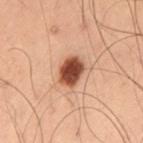biopsy status: imaged on a skin check; not biopsied
tile lighting: cross-polarized
imaging modality: total-body-photography crop, ~15 mm field of view
subject: male, roughly 55 years of age
lesion size: about 3.5 mm
location: the left thigh